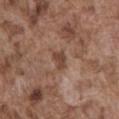The lesion was tiled from a total-body skin photograph and was not biopsied.
The lesion is on the abdomen.
Captured under white-light illumination.
This image is a 15 mm lesion crop taken from a total-body photograph.
The subject is a male about 75 years old.
About 2.5 mm across.